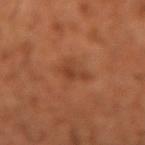No biopsy was performed on this lesion — it was imaged during a full skin examination and was not determined to be concerning. The lesion-visualizer software estimated a lesion area of about 4 mm², a shape eccentricity near 0.6, and a shape-asymmetry score of about 0.45 (0 = symmetric). A 15 mm close-up extracted from a 3D total-body photography capture. A male subject about 60 years old. The lesion is on the left forearm. About 2.5 mm across.